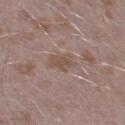– biopsy status — total-body-photography surveillance lesion; no biopsy
– illumination — white-light illumination
– subject — male, in their 40s
– body site — the left lower leg
– lesion size — ~3 mm (longest diameter)
– image-analysis metrics — about 7 CIELAB-L* units darker than the surrounding skin and a normalized lesion–skin contrast near 6; internal color variation of about 2.5 on a 0–10 scale and radial color variation of about 1; a classifier nevus-likeness of about 0/100 and a detector confidence of about 100 out of 100 that the crop contains a lesion
– image source — total-body-photography crop, ~15 mm field of view